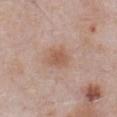Q: Was this lesion biopsied?
A: catalogued during a skin exam; not biopsied
Q: Lesion size?
A: ≈3 mm
Q: How was this image acquired?
A: 15 mm crop, total-body photography
Q: What are the patient's age and sex?
A: male, approximately 55 years of age
Q: What is the anatomic site?
A: the abdomen
Q: How was the tile lit?
A: white-light
Q: What did automated image analysis measure?
A: a lesion area of about 5 mm², an eccentricity of roughly 0.65, and two-axis asymmetry of about 0.3; a border-irregularity index near 2.5/10, a color-variation rating of about 1.5/10, and a peripheral color-asymmetry measure near 0.5; lesion-presence confidence of about 100/100